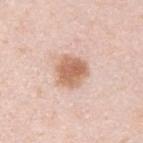Clinical impression:
Imaged during a routine full-body skin examination; the lesion was not biopsied and no histopathology is available.
Image and clinical context:
The lesion is located on the upper back. A region of skin cropped from a whole-body photographic capture, roughly 15 mm wide. A male subject approximately 35 years of age. About 3.5 mm across. Imaged with white-light lighting. The total-body-photography lesion software estimated a lesion color around L≈64 a*≈21 b*≈31 in CIELAB and roughly 13 lightness units darker than nearby skin. And it measured a nevus-likeness score of about 95/100 and a lesion-detection confidence of about 100/100.